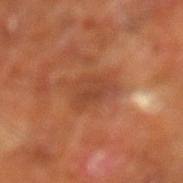Findings:
* follow-up — total-body-photography surveillance lesion; no biopsy
* size — ≈3.5 mm
* subject — male, aged around 65
* tile lighting — cross-polarized
* acquisition — ~15 mm crop, total-body skin-cancer survey
* body site — the leg